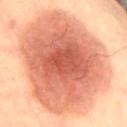{"biopsy_status": "not biopsied; imaged during a skin examination", "patient": {"sex": "male", "age_approx": 55}, "lesion_size": {"long_diameter_mm_approx": 13.5}, "site": "lower back", "image": {"source": "total-body photography crop", "field_of_view_mm": 15}}